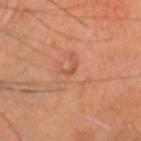Clinical impression:
No biopsy was performed on this lesion — it was imaged during a full skin examination and was not determined to be concerning.
Background:
The tile uses cross-polarized illumination. Located on the head or neck. A lesion tile, about 15 mm wide, cut from a 3D total-body photograph. A male patient in their 60s.